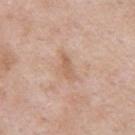{"biopsy_status": "not biopsied; imaged during a skin examination", "site": "left upper arm", "patient": {"sex": "male", "age_approx": 55}, "lighting": "white-light", "lesion_size": {"long_diameter_mm_approx": 3.0}, "image": {"source": "total-body photography crop", "field_of_view_mm": 15}}